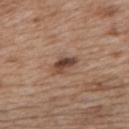Q: Was this lesion biopsied?
A: catalogued during a skin exam; not biopsied
Q: Where on the body is the lesion?
A: the back
Q: What are the patient's age and sex?
A: female, aged approximately 40
Q: What is the imaging modality?
A: total-body-photography crop, ~15 mm field of view
Q: Automated lesion metrics?
A: border irregularity of about 3 on a 0–10 scale, a within-lesion color-variation index near 7/10, and peripheral color asymmetry of about 2.5
Q: How was the tile lit?
A: white-light illumination